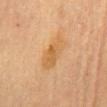The lesion was tiled from a total-body skin photograph and was not biopsied.